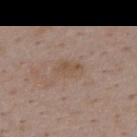{
  "biopsy_status": "not biopsied; imaged during a skin examination"
}